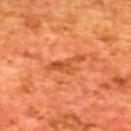biopsy_status: not biopsied; imaged during a skin examination
lesion_size:
  long_diameter_mm_approx: 4.5
image:
  source: total-body photography crop
  field_of_view_mm: 15
site: upper back
patient:
  sex: male
  age_approx: 65
automated_metrics:
  area_mm2_approx: 5.0
  eccentricity: 0.9
  shape_asymmetry: 0.6
  cielab_L: 48
  cielab_a: 32
  cielab_b: 41
  vs_skin_contrast_norm: 6.5
  nevus_likeness_0_100: 0
  lesion_detection_confidence_0_100: 95